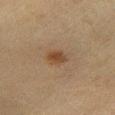follow-up = no biopsy performed (imaged during a skin exam) | acquisition = 15 mm crop, total-body photography | lesion size = about 2.5 mm | patient = male, about 65 years old | automated lesion analysis = a footprint of about 3.5 mm² and a shape eccentricity near 0.7; an average lesion color of about L≈35 a*≈15 b*≈27 (CIELAB), roughly 8 lightness units darker than nearby skin, and a normalized border contrast of about 8; a classifier nevus-likeness of about 95/100 and a lesion-detection confidence of about 100/100 | illumination = cross-polarized illumination | location = the right lower leg.